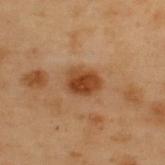Case summary:
- location: the upper back
- subject: male, aged 53–57
- image source: ~15 mm crop, total-body skin-cancer survey